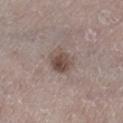| field | value |
|---|---|
| biopsy status | no biopsy performed (imaged during a skin exam) |
| lighting | white-light illumination |
| location | the leg |
| image source | 15 mm crop, total-body photography |
| size | ≈3 mm |
| automated lesion analysis | an automated nevus-likeness rating near 90 out of 100 and a detector confidence of about 100 out of 100 that the crop contains a lesion |
| subject | male, roughly 65 years of age |